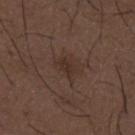notes: no biopsy performed (imaged during a skin exam) | acquisition: ~15 mm crop, total-body skin-cancer survey | site: the mid back | patient: male, roughly 50 years of age.